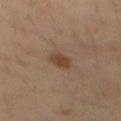• biopsy status — imaged on a skin check; not biopsied
• diameter — ≈3 mm
• acquisition — 15 mm crop, total-body photography
• subject — male, roughly 30 years of age
• location — the mid back